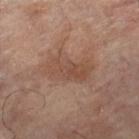| key | value |
|---|---|
| biopsy status | no biopsy performed (imaged during a skin exam) |
| illumination | cross-polarized |
| anatomic site | the left leg |
| diameter | ≈5.5 mm |
| patient | female, about 80 years old |
| image | total-body-photography crop, ~15 mm field of view |